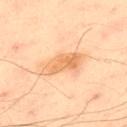Impression: The lesion was photographed on a routine skin check and not biopsied; there is no pathology result. Context: Located on the upper back. Imaged with cross-polarized lighting. Automated tile analysis of the lesion measured an area of roughly 9.5 mm², an outline eccentricity of about 0.9 (0 = round, 1 = elongated), and two-axis asymmetry of about 0.25. The software also gave a border-irregularity rating of about 3/10. And it measured a nevus-likeness score of about 10/100 and lesion-presence confidence of about 100/100. The patient is a male in their 50s. A 15 mm close-up extracted from a 3D total-body photography capture. About 5 mm across.